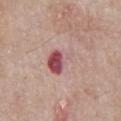{"biopsy_status": "not biopsied; imaged during a skin examination", "image": {"source": "total-body photography crop", "field_of_view_mm": 15}, "patient": {"sex": "male", "age_approx": 65}, "site": "chest"}